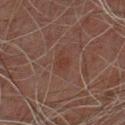<case>
<lighting>cross-polarized</lighting>
<site>chest</site>
<lesion_size>
  <long_diameter_mm_approx>2.5</long_diameter_mm_approx>
</lesion_size>
<patient>
  <sex>male</sex>
  <age_approx>45</age_approx>
</patient>
<image>
  <source>total-body photography crop</source>
  <field_of_view_mm>15</field_of_view_mm>
</image>
</case>